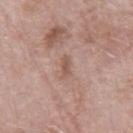The lesion was tiled from a total-body skin photograph and was not biopsied.
Approximately 2.5 mm at its widest.
Captured under white-light illumination.
The lesion is on the right thigh.
A roughly 15 mm field-of-view crop from a total-body skin photograph.
A female subject aged around 75.